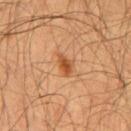notes: imaged on a skin check; not biopsied
patient: male, aged 58–62
size: ~3 mm (longest diameter)
site: the chest
image: total-body-photography crop, ~15 mm field of view
illumination: cross-polarized illumination